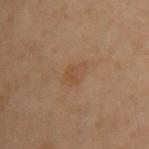Located on the arm. A close-up tile cropped from a whole-body skin photograph, about 15 mm across. The lesion's longest dimension is about 3 mm. The total-body-photography lesion software estimated a lesion color around L≈42 a*≈16 b*≈29 in CIELAB, about 5 CIELAB-L* units darker than the surrounding skin, and a lesion-to-skin contrast of about 5 (normalized; higher = more distinct). The analysis additionally found lesion-presence confidence of about 100/100. The patient is a female aged around 60.